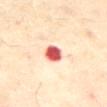This image is a 15 mm lesion crop taken from a total-body photograph. Captured under cross-polarized illumination. A male subject, about 70 years old. The lesion is located on the abdomen. About 3 mm across. An algorithmic analysis of the crop reported an outline eccentricity of about 0.6 (0 = round, 1 = elongated) and two-axis asymmetry of about 0.2. The software also gave about 24 CIELAB-L* units darker than the surrounding skin and a normalized lesion–skin contrast near 13. The analysis additionally found a border-irregularity rating of about 1.5/10 and a peripheral color-asymmetry measure near 1.5. The software also gave a nevus-likeness score of about 0/100.